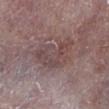Impression: Recorded during total-body skin imaging; not selected for excision or biopsy. Acquisition and patient details: A lesion tile, about 15 mm wide, cut from a 3D total-body photograph. A male patient, about 75 years old. Measured at roughly 6 mm in maximum diameter. The lesion is located on the leg.